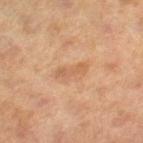biopsy_status: not biopsied; imaged during a skin examination
lesion_size:
  long_diameter_mm_approx: 3.5
site: leg
image:
  source: total-body photography crop
  field_of_view_mm: 15
lighting: cross-polarized
patient:
  sex: female
  age_approx: 60